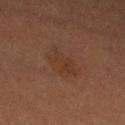The lesion was tiled from a total-body skin photograph and was not biopsied.
A 15 mm close-up extracted from a 3D total-body photography capture.
The lesion is located on the left thigh.
The subject is a female roughly 55 years of age.
Measured at roughly 4 mm in maximum diameter.
Automated image analysis of the tile measured an area of roughly 10 mm², a shape eccentricity near 0.7, and a shape-asymmetry score of about 0.2 (0 = symmetric). It also reported a nevus-likeness score of about 20/100 and a lesion-detection confidence of about 100/100.
The tile uses cross-polarized illumination.